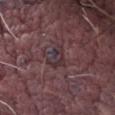  biopsy_status: not biopsied; imaged during a skin examination
  lesion_size:
    long_diameter_mm_approx: 3.0
  lighting: white-light
  site: abdomen
  image:
    source: total-body photography crop
    field_of_view_mm: 15
  patient:
    sex: male
    age_approx: 70